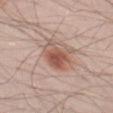Clinical impression: No biopsy was performed on this lesion — it was imaged during a full skin examination and was not determined to be concerning. Context: The lesion is on the left thigh. Longest diameter approximately 4 mm. This image is a 15 mm lesion crop taken from a total-body photograph. A male subject, aged around 25.